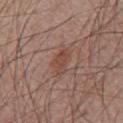biopsy status: no biopsy performed (imaged during a skin exam) | illumination: white-light illumination | anatomic site: the chest | image source: ~15 mm tile from a whole-body skin photo | diameter: about 3.5 mm | automated metrics: an outline eccentricity of about 0.85 (0 = round, 1 = elongated); a mean CIELAB color near L≈46 a*≈20 b*≈26, a lesion–skin lightness drop of about 7, and a lesion-to-skin contrast of about 6.5 (normalized; higher = more distinct) | subject: male, roughly 70 years of age.